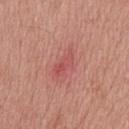Assessment: Imaged during a routine full-body skin examination; the lesion was not biopsied and no histopathology is available. Context: The lesion is located on the upper back. A lesion tile, about 15 mm wide, cut from a 3D total-body photograph. The recorded lesion diameter is about 4 mm. A female patient, roughly 40 years of age.